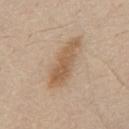The lesion was photographed on a routine skin check and not biopsied; there is no pathology result. The lesion is located on the chest. The lesion's longest dimension is about 7 mm. Automated tile analysis of the lesion measured a footprint of about 13 mm², a shape eccentricity near 0.95, and a shape-asymmetry score of about 0.25 (0 = symmetric). The analysis additionally found an automated nevus-likeness rating near 50 out of 100 and a detector confidence of about 100 out of 100 that the crop contains a lesion. A male patient aged 78 to 82. Cropped from a whole-body photographic skin survey; the tile spans about 15 mm. The tile uses white-light illumination.